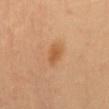workup: total-body-photography surveillance lesion; no biopsy | image: ~15 mm crop, total-body skin-cancer survey | anatomic site: the mid back | illumination: cross-polarized | patient: female, approximately 60 years of age | lesion diameter: about 3 mm.